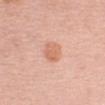Imaged during a routine full-body skin examination; the lesion was not biopsied and no histopathology is available. A close-up tile cropped from a whole-body skin photograph, about 15 mm across. The patient is a female aged around 40. Measured at roughly 2.5 mm in maximum diameter. On the left upper arm.